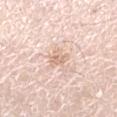biopsy_status: not biopsied; imaged during a skin examination
site: left lower leg
image:
  source: total-body photography crop
  field_of_view_mm: 15
automated_metrics:
  cielab_L: 72
  cielab_a: 18
  cielab_b: 29
  vs_skin_darker_L: 9.0
  vs_skin_contrast_norm: 5.5
  border_irregularity_0_10: 4.0
  color_variation_0_10: 2.5
lesion_size:
  long_diameter_mm_approx: 2.5
lighting: white-light
patient:
  sex: female
  age_approx: 45